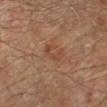A male subject in their mid-60s. The lesion is located on the right lower leg. The lesion-visualizer software estimated a lesion area of about 4 mm², a shape eccentricity near 0.85, and a shape-asymmetry score of about 0.5 (0 = symmetric). And it measured an automated nevus-likeness rating near 5 out of 100 and lesion-presence confidence of about 100/100. A region of skin cropped from a whole-body photographic capture, roughly 15 mm wide. The recorded lesion diameter is about 3 mm. The tile uses cross-polarized illumination.